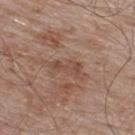Background: A lesion tile, about 15 mm wide, cut from a 3D total-body photograph. The lesion-visualizer software estimated a lesion color around L≈47 a*≈18 b*≈27 in CIELAB, roughly 7 lightness units darker than nearby skin, and a normalized lesion–skin contrast near 6. And it measured an automated nevus-likeness rating near 0 out of 100 and a lesion-detection confidence of about 100/100. The lesion is on the upper back. The tile uses white-light illumination. The lesion's longest dimension is about 3.5 mm. A male patient aged approximately 55.